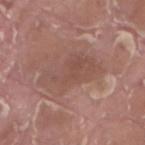Assessment:
No biopsy was performed on this lesion — it was imaged during a full skin examination and was not determined to be concerning.
Background:
A close-up tile cropped from a whole-body skin photograph, about 15 mm across. A male patient in their 40s. The lesion is on the right thigh.